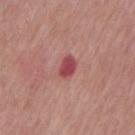Recorded during total-body skin imaging; not selected for excision or biopsy. This is a white-light tile. An algorithmic analysis of the crop reported an automated nevus-likeness rating near 5 out of 100 and a detector confidence of about 100 out of 100 that the crop contains a lesion. A region of skin cropped from a whole-body photographic capture, roughly 15 mm wide. A male subject, in their mid- to late 60s. On the chest. The recorded lesion diameter is about 3 mm.